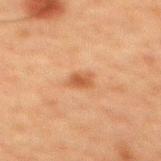notes: catalogued during a skin exam; not biopsied
size: ≈2.5 mm
illumination: cross-polarized illumination
subject: male, aged around 60
acquisition: 15 mm crop, total-body photography
body site: the mid back